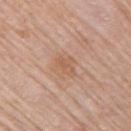notes=no biopsy performed (imaged during a skin exam); site=the right upper arm; subject=female, aged approximately 65; lighting=white-light; acquisition=~15 mm tile from a whole-body skin photo; size=~3 mm (longest diameter).